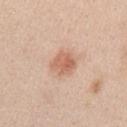This lesion was catalogued during total-body skin photography and was not selected for biopsy. Cropped from a whole-body photographic skin survey; the tile spans about 15 mm. Automated tile analysis of the lesion measured a lesion area of about 7 mm² and an outline eccentricity of about 0.5 (0 = round, 1 = elongated). Imaged with white-light lighting. Longest diameter approximately 3 mm. On the right upper arm. A female subject aged 38–42.